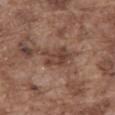<record>
<biopsy_status>not biopsied; imaged during a skin examination</biopsy_status>
<automated_metrics>
  <cielab_L>42</cielab_L>
  <cielab_a>19</cielab_a>
  <cielab_b>25</cielab_b>
  <vs_skin_darker_L>9.0</vs_skin_darker_L>
  <vs_skin_contrast_norm>7.5</vs_skin_contrast_norm>
  <nevus_likeness_0_100>5</nevus_likeness_0_100>
  <lesion_detection_confidence_0_100>95</lesion_detection_confidence_0_100>
</automated_metrics>
<lesion_size>
  <long_diameter_mm_approx>3.5</long_diameter_mm_approx>
</lesion_size>
<site>abdomen</site>
<image>
  <source>total-body photography crop</source>
  <field_of_view_mm>15</field_of_view_mm>
</image>
<lighting>white-light</lighting>
<patient>
  <sex>male</sex>
  <age_approx>75</age_approx>
</patient>
</record>